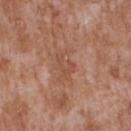  biopsy_status: not biopsied; imaged during a skin examination
  image:
    source: total-body photography crop
    field_of_view_mm: 15
  lesion_size:
    long_diameter_mm_approx: 3.0
  patient:
    sex: male
    age_approx: 45
  site: upper back
  lighting: white-light